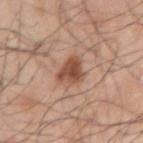notes: no biopsy performed (imaged during a skin exam)
lighting: white-light
subject: male, roughly 65 years of age
automated metrics: a lesion color around L≈50 a*≈22 b*≈30 in CIELAB, about 13 CIELAB-L* units darker than the surrounding skin, and a normalized border contrast of about 9.5; a nevus-likeness score of about 90/100 and a detector confidence of about 100 out of 100 that the crop contains a lesion
diameter: ~3 mm (longest diameter)
site: the right upper arm
image source: ~15 mm crop, total-body skin-cancer survey